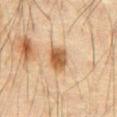This lesion was catalogued during total-body skin photography and was not selected for biopsy.
This image is a 15 mm lesion crop taken from a total-body photograph.
Automated image analysis of the tile measured an eccentricity of roughly 0.65 and a symmetry-axis asymmetry near 0.2. The software also gave an automated nevus-likeness rating near 95 out of 100 and lesion-presence confidence of about 100/100.
About 3.5 mm across.
The lesion is located on the abdomen.
A male subject, about 65 years old.